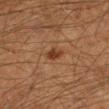Q: Was this lesion biopsied?
A: imaged on a skin check; not biopsied
Q: What did automated image analysis measure?
A: an area of roughly 3 mm², an outline eccentricity of about 0.8 (0 = round, 1 = elongated), and a symmetry-axis asymmetry near 0.3
Q: How was this image acquired?
A: total-body-photography crop, ~15 mm field of view
Q: How was the tile lit?
A: cross-polarized illumination
Q: How large is the lesion?
A: ~2.5 mm (longest diameter)
Q: Patient demographics?
A: male, in their 50s
Q: Lesion location?
A: the left forearm